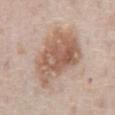biopsy status: imaged on a skin check; not biopsied | acquisition: 15 mm crop, total-body photography | lesion size: ≈7.5 mm | automated metrics: an average lesion color of about L≈58 a*≈18 b*≈28 (CIELAB) and a normalized border contrast of about 8; a nevus-likeness score of about 50/100 and lesion-presence confidence of about 100/100 | lighting: white-light | body site: the abdomen | subject: male, aged 73 to 77.